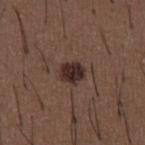Findings:
* biopsy status · total-body-photography surveillance lesion; no biopsy
* patient · male, roughly 50 years of age
* illumination · white-light
* acquisition · total-body-photography crop, ~15 mm field of view
* size · ≈2.5 mm
* body site · the abdomen